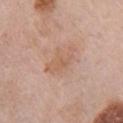Clinical impression: This lesion was catalogued during total-body skin photography and was not selected for biopsy. Image and clinical context: The lesion is located on the right upper arm. Automated image analysis of the tile measured a lesion area of about 7.5 mm² and a symmetry-axis asymmetry near 0.3. The analysis additionally found an average lesion color of about L≈60 a*≈20 b*≈30 (CIELAB) and about 6 CIELAB-L* units darker than the surrounding skin. The tile uses white-light illumination. The subject is a female in their mid-60s. A 15 mm crop from a total-body photograph taken for skin-cancer surveillance.